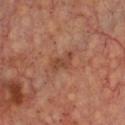imaging modality = ~15 mm crop, total-body skin-cancer survey | diameter = about 3.5 mm | location = the chest | patient = aged approximately 65.